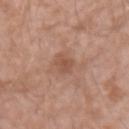Impression:
The lesion was tiled from a total-body skin photograph and was not biopsied.
Context:
The total-body-photography lesion software estimated an eccentricity of roughly 0.65 and a symmetry-axis asymmetry near 0.2. The software also gave a lesion–skin lightness drop of about 8 and a lesion-to-skin contrast of about 6 (normalized; higher = more distinct). It also reported an automated nevus-likeness rating near 0 out of 100 and a lesion-detection confidence of about 100/100. Measured at roughly 3 mm in maximum diameter. A region of skin cropped from a whole-body photographic capture, roughly 15 mm wide. The lesion is located on the right upper arm. The patient is a male in their 60s. Captured under white-light illumination.